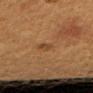<case>
<automated_metrics>
  <cielab_L>36</cielab_L>
  <cielab_a>16</cielab_a>
  <cielab_b>29</cielab_b>
  <vs_skin_darker_L>5.0</vs_skin_darker_L>
  <vs_skin_contrast_norm>4.5</vs_skin_contrast_norm>
  <border_irregularity_0_10>3.0</border_irregularity_0_10>
  <color_variation_0_10>2.0</color_variation_0_10>
  <peripheral_color_asymmetry>1.0</peripheral_color_asymmetry>
  <lesion_detection_confidence_0_100>100</lesion_detection_confidence_0_100>
</automated_metrics>
<lighting>cross-polarized</lighting>
<image>
  <source>total-body photography crop</source>
  <field_of_view_mm>15</field_of_view_mm>
</image>
<lesion_size>
  <long_diameter_mm_approx>2.5</long_diameter_mm_approx>
</lesion_size>
<site>arm</site>
<patient>
  <sex>female</sex>
  <age_approx>40</age_approx>
</patient>
</case>